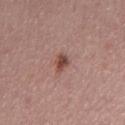  biopsy_status: not biopsied; imaged during a skin examination
  image:
    source: total-body photography crop
    field_of_view_mm: 15
  lighting: white-light
  lesion_size:
    long_diameter_mm_approx: 2.5
  patient:
    sex: male
    age_approx: 40
  site: chest
  automated_metrics:
    area_mm2_approx: 3.5
    eccentricity: 0.75
    shape_asymmetry: 0.25